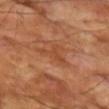anatomic site: the left upper arm
image source: total-body-photography crop, ~15 mm field of view
patient: male, aged 68 to 72